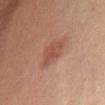follow-up = imaged on a skin check; not biopsied | site = the chest | image = ~15 mm tile from a whole-body skin photo | patient = female, in their 60s.